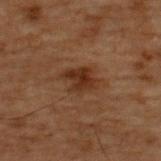Notes:
• biopsy status: catalogued during a skin exam; not biopsied
• location: the upper back
• subject: male, approximately 70 years of age
• lesion size: about 4 mm
• imaging modality: ~15 mm tile from a whole-body skin photo
• lighting: cross-polarized illumination